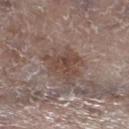<case>
  <biopsy_status>not biopsied; imaged during a skin examination</biopsy_status>
  <patient>
    <sex>female</sex>
    <age_approx>85+</age_approx>
  </patient>
  <automated_metrics>
    <area_mm2_approx>13.0</area_mm2_approx>
    <eccentricity>0.5</eccentricity>
    <cielab_L>46</cielab_L>
    <cielab_a>16</cielab_a>
    <cielab_b>23</cielab_b>
    <vs_skin_darker_L>8.0</vs_skin_darker_L>
    <vs_skin_contrast_norm>7.0</vs_skin_contrast_norm>
  </automated_metrics>
  <image>
    <source>total-body photography crop</source>
    <field_of_view_mm>15</field_of_view_mm>
  </image>
  <site>leg</site>
  <lighting>white-light</lighting>
  <lesion_size>
    <long_diameter_mm_approx>4.5</long_diameter_mm_approx>
  </lesion_size>
</case>